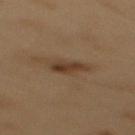Captured during whole-body skin photography for melanoma surveillance; the lesion was not biopsied.
From the mid back.
The patient is a male aged 53–57.
A lesion tile, about 15 mm wide, cut from a 3D total-body photograph.
An algorithmic analysis of the crop reported a mean CIELAB color near L≈29 a*≈13 b*≈24, a lesion–skin lightness drop of about 7, and a normalized border contrast of about 8.